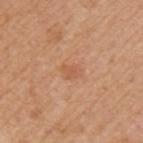Part of a total-body skin-imaging series; this lesion was reviewed on a skin check and was not flagged for biopsy. Automated tile analysis of the lesion measured an area of roughly 4 mm² and a shape eccentricity near 0.7. The analysis additionally found an automated nevus-likeness rating near 0 out of 100 and a detector confidence of about 100 out of 100 that the crop contains a lesion. The recorded lesion diameter is about 2.5 mm. A roughly 15 mm field-of-view crop from a total-body skin photograph. Located on the left upper arm. The patient is a male roughly 65 years of age.